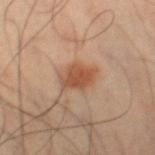Captured during whole-body skin photography for melanoma surveillance; the lesion was not biopsied. The total-body-photography lesion software estimated an outline eccentricity of about 0.75 (0 = round, 1 = elongated) and a shape-asymmetry score of about 0.2 (0 = symmetric). And it measured a mean CIELAB color near L≈50 a*≈22 b*≈32, about 11 CIELAB-L* units darker than the surrounding skin, and a lesion-to-skin contrast of about 8 (normalized; higher = more distinct). It also reported a border-irregularity index near 2.5/10, a within-lesion color-variation index near 3/10, and a peripheral color-asymmetry measure near 1. The analysis additionally found a classifier nevus-likeness of about 95/100 and a lesion-detection confidence of about 100/100. A 15 mm close-up extracted from a 3D total-body photography capture. The subject is a male aged 38–42. On the right thigh. Measured at roughly 3.5 mm in maximum diameter. Imaged with cross-polarized lighting.